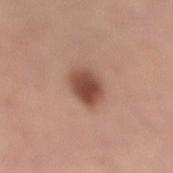Findings:
* workup · catalogued during a skin exam; not biopsied
* illumination · white-light
* patient · female, about 55 years old
* body site · the right lower leg
* image · 15 mm crop, total-body photography